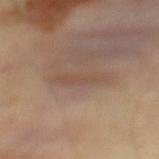Located on the mid back.
The subject is a male aged 38 to 42.
A 15 mm close-up tile from a total-body photography series done for melanoma screening.
This is a cross-polarized tile.
About 5 mm across.